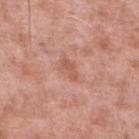biopsy status=no biopsy performed (imaged during a skin exam) | location=the right lower leg | image source=~15 mm crop, total-body skin-cancer survey | subject=male, roughly 55 years of age | image-analysis metrics=a shape eccentricity near 0.9 and two-axis asymmetry of about 0.3; a color-variation rating of about 0/10; a nevus-likeness score of about 0/100 and lesion-presence confidence of about 100/100.